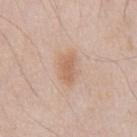Q: Was this lesion biopsied?
A: catalogued during a skin exam; not biopsied
Q: How large is the lesion?
A: about 3.5 mm
Q: What is the anatomic site?
A: the chest
Q: What is the imaging modality?
A: ~15 mm crop, total-body skin-cancer survey
Q: Who is the patient?
A: male, about 45 years old
Q: Automated lesion metrics?
A: a footprint of about 5 mm², a shape eccentricity near 0.85, and a shape-asymmetry score of about 0.3 (0 = symmetric); a lesion color around L≈62 a*≈19 b*≈31 in CIELAB and about 9 CIELAB-L* units darker than the surrounding skin; a border-irregularity index near 3/10, internal color variation of about 1.5 on a 0–10 scale, and a peripheral color-asymmetry measure near 0.5; an automated nevus-likeness rating near 55 out of 100 and a detector confidence of about 100 out of 100 that the crop contains a lesion